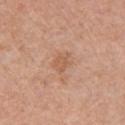Clinical impression: This lesion was catalogued during total-body skin photography and was not selected for biopsy. Background: From the left upper arm. A female subject aged 58–62. This image is a 15 mm lesion crop taken from a total-body photograph. An algorithmic analysis of the crop reported a mean CIELAB color near L≈58 a*≈21 b*≈33, roughly 7 lightness units darker than nearby skin, and a lesion-to-skin contrast of about 5 (normalized; higher = more distinct). The analysis additionally found an automated nevus-likeness rating near 0 out of 100 and lesion-presence confidence of about 100/100. Approximately 2.5 mm at its widest. The tile uses white-light illumination.